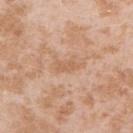notes: catalogued during a skin exam; not biopsied | acquisition: ~15 mm crop, total-body skin-cancer survey | size: ≈2.5 mm | TBP lesion metrics: a classifier nevus-likeness of about 0/100 and a lesion-detection confidence of about 100/100 | subject: female, in their mid- to late 20s | anatomic site: the right upper arm | illumination: white-light.